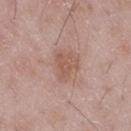notes=no biopsy performed (imaged during a skin exam); size=~3.5 mm (longest diameter); patient=male, roughly 50 years of age; body site=the right thigh; tile lighting=white-light illumination; imaging modality=15 mm crop, total-body photography.